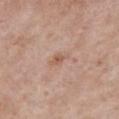Clinical summary:
This is a white-light tile. From the chest. The subject is a female about 85 years old or older. The total-body-photography lesion software estimated a lesion color around L≈57 a*≈20 b*≈29 in CIELAB, about 8 CIELAB-L* units darker than the surrounding skin, and a lesion-to-skin contrast of about 6 (normalized; higher = more distinct). The analysis additionally found lesion-presence confidence of about 100/100. Cropped from a whole-body photographic skin survey; the tile spans about 15 mm.